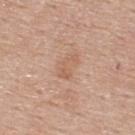Context: A lesion tile, about 15 mm wide, cut from a 3D total-body photograph. A female patient in their mid- to late 60s. On the upper back.